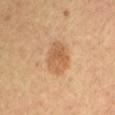• follow-up · total-body-photography surveillance lesion; no biopsy
• subject · female, aged 63–67
• imaging modality · total-body-photography crop, ~15 mm field of view
• site · the mid back